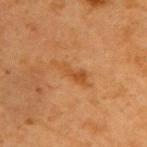  biopsy_status: not biopsied; imaged during a skin examination
  site: right upper arm
  patient:
    sex: female
    age_approx: 40
  image:
    source: total-body photography crop
    field_of_view_mm: 15